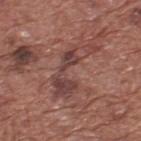{"biopsy_status": "not biopsied; imaged during a skin examination", "patient": {"sex": "male", "age_approx": 75}, "image": {"source": "total-body photography crop", "field_of_view_mm": 15}, "lighting": "white-light", "site": "upper back", "lesion_size": {"long_diameter_mm_approx": 7.5}}